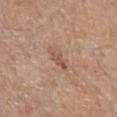Q: Was this lesion biopsied?
A: total-body-photography surveillance lesion; no biopsy
Q: How large is the lesion?
A: ~3 mm (longest diameter)
Q: What is the imaging modality?
A: ~15 mm tile from a whole-body skin photo
Q: Where on the body is the lesion?
A: the chest
Q: What are the patient's age and sex?
A: male, aged approximately 65